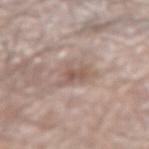Clinical impression: Part of a total-body skin-imaging series; this lesion was reviewed on a skin check and was not flagged for biopsy. Acquisition and patient details: On the left arm. A 15 mm crop from a total-body photograph taken for skin-cancer surveillance. A male patient, about 80 years old. Captured under white-light illumination. Longest diameter approximately 3.5 mm.